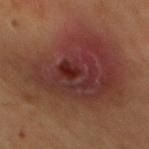Captured during whole-body skin photography for melanoma surveillance; the lesion was not biopsied. The lesion-visualizer software estimated an area of roughly 85 mm² and a symmetry-axis asymmetry near 0.3. It also reported a nevus-likeness score of about 0/100 and a lesion-detection confidence of about 100/100. Located on the back. Cropped from a total-body skin-imaging series; the visible field is about 15 mm. A male subject, aged around 60.